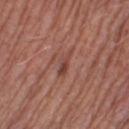This lesion was catalogued during total-body skin photography and was not selected for biopsy.